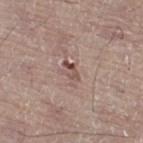  biopsy_status: not biopsied; imaged during a skin examination
  lighting: white-light
  site: right leg
  image:
    source: total-body photography crop
    field_of_view_mm: 15
  patient:
    sex: male
    age_approx: 80